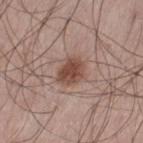Q: Was a biopsy performed?
A: no biopsy performed (imaged during a skin exam)
Q: Who is the patient?
A: male, aged 58 to 62
Q: Lesion location?
A: the left thigh
Q: What kind of image is this?
A: ~15 mm tile from a whole-body skin photo